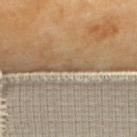Automated tile analysis of the lesion measured a border-irregularity index near 3/10 and internal color variation of about 0 on a 0–10 scale. It also reported a nevus-likeness score of about 0/100 and a lesion-detection confidence of about 0/100. Located on the upper back. About 1 mm across. A lesion tile, about 15 mm wide, cut from a 3D total-body photograph. The subject is a female aged approximately 70. Imaged with cross-polarized lighting.